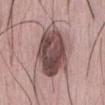Assessment:
Recorded during total-body skin imaging; not selected for excision or biopsy.
Context:
Cropped from a whole-body photographic skin survey; the tile spans about 15 mm. A male patient, approximately 30 years of age. The tile uses white-light illumination. The lesion is located on the abdomen. About 7 mm across.